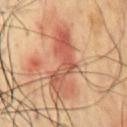Case summary:
* imaging modality — ~15 mm tile from a whole-body skin photo
* patient — male, in their 70s
* site — the chest
* lighting — cross-polarized illumination